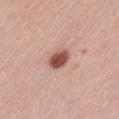Imaged during a routine full-body skin examination; the lesion was not biopsied and no histopathology is available.